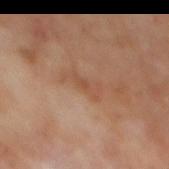Q: Is there a histopathology result?
A: imaged on a skin check; not biopsied
Q: How large is the lesion?
A: ~4 mm (longest diameter)
Q: How was this image acquired?
A: total-body-photography crop, ~15 mm field of view
Q: What is the anatomic site?
A: the mid back
Q: Patient demographics?
A: male, approximately 70 years of age
Q: What lighting was used for the tile?
A: cross-polarized